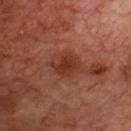Case summary:
– notes · no biopsy performed (imaged during a skin exam)
– body site · the front of the torso
– automated lesion analysis · an area of roughly 10 mm², an outline eccentricity of about 0.65 (0 = round, 1 = elongated), and a shape-asymmetry score of about 0.15 (0 = symmetric); an automated nevus-likeness rating near 45 out of 100
– subject · male, about 70 years old
– image · 15 mm crop, total-body photography
– size · ~4.5 mm (longest diameter)
– illumination · cross-polarized illumination